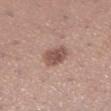Impression:
Imaged during a routine full-body skin examination; the lesion was not biopsied and no histopathology is available.
Background:
The lesion-visualizer software estimated border irregularity of about 1.5 on a 0–10 scale, internal color variation of about 3 on a 0–10 scale, and a peripheral color-asymmetry measure near 1. This image is a 15 mm lesion crop taken from a total-body photograph. On the right lower leg. The recorded lesion diameter is about 3 mm. The tile uses white-light illumination. The patient is a female roughly 30 years of age.